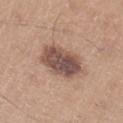Imaged during a routine full-body skin examination; the lesion was not biopsied and no histopathology is available.
The lesion-visualizer software estimated an area of roughly 16 mm², an eccentricity of roughly 0.75, and a symmetry-axis asymmetry near 0.15. It also reported a mean CIELAB color near L≈50 a*≈18 b*≈23 and a normalized lesion–skin contrast near 10. The analysis additionally found border irregularity of about 2 on a 0–10 scale, a within-lesion color-variation index near 5.5/10, and peripheral color asymmetry of about 2. The software also gave a detector confidence of about 100 out of 100 that the crop contains a lesion.
Approximately 5.5 mm at its widest.
A male subject, aged 38–42.
From the right lower leg.
A lesion tile, about 15 mm wide, cut from a 3D total-body photograph.